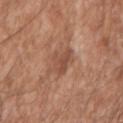No biopsy was performed on this lesion — it was imaged during a full skin examination and was not determined to be concerning.
On the left upper arm.
A lesion tile, about 15 mm wide, cut from a 3D total-body photograph.
A male patient roughly 60 years of age.